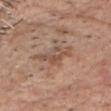Imaged during a routine full-body skin examination; the lesion was not biopsied and no histopathology is available. Cropped from a whole-body photographic skin survey; the tile spans about 15 mm. The lesion is located on the chest. A male subject about 80 years old. An algorithmic analysis of the crop reported a footprint of about 8.5 mm², an eccentricity of roughly 0.85, and a shape-asymmetry score of about 0.3 (0 = symmetric). The analysis additionally found a border-irregularity index near 4.5/10 and internal color variation of about 4 on a 0–10 scale. It also reported an automated nevus-likeness rating near 0 out of 100 and lesion-presence confidence of about 95/100.